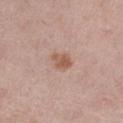Context: An algorithmic analysis of the crop reported a footprint of about 4 mm², a shape eccentricity near 0.75, and a symmetry-axis asymmetry near 0.2. A 15 mm close-up extracted from a 3D total-body photography capture. The recorded lesion diameter is about 2.5 mm. Imaged with white-light lighting. A female subject about 40 years old. Located on the right thigh.